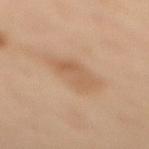subject — female, aged 38–42
location — the left leg
diameter — ≈5 mm
tile lighting — cross-polarized illumination
acquisition — ~15 mm crop, total-body skin-cancer survey
TBP lesion metrics — a border-irregularity rating of about 3.5/10; a lesion-detection confidence of about 100/100